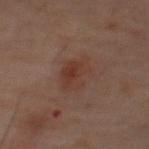Findings:
* workup — no biopsy performed (imaged during a skin exam)
* patient — male, aged 58–62
* size — ≈4.5 mm
* anatomic site — the chest
* lighting — cross-polarized illumination
* image — ~15 mm tile from a whole-body skin photo